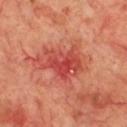Part of a total-body skin-imaging series; this lesion was reviewed on a skin check and was not flagged for biopsy.
The tile uses cross-polarized illumination.
The recorded lesion diameter is about 4.5 mm.
A 15 mm close-up extracted from a 3D total-body photography capture.
From the chest.
The lesion-visualizer software estimated a lesion area of about 12 mm². It also reported a mean CIELAB color near L≈47 a*≈38 b*≈31 and about 10 CIELAB-L* units darker than the surrounding skin. It also reported a nevus-likeness score of about 0/100 and lesion-presence confidence of about 100/100.
A male subject, aged 68–72.